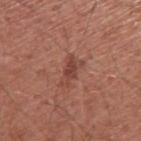Recorded during total-body skin imaging; not selected for excision or biopsy. The lesion is on the right upper arm. Longest diameter approximately 3.5 mm. A male subject, aged 58 to 62. A 15 mm close-up extracted from a 3D total-body photography capture.